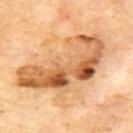About 11 mm across. A male subject aged around 70. The lesion-visualizer software estimated an average lesion color of about L≈61 a*≈22 b*≈41 (CIELAB) and roughly 15 lightness units darker than nearby skin. The software also gave a border-irregularity index near 6.5/10, a within-lesion color-variation index near 10/10, and a peripheral color-asymmetry measure near 4.5. The analysis additionally found a nevus-likeness score of about 25/100 and a detector confidence of about 55 out of 100 that the crop contains a lesion. This image is a 15 mm lesion crop taken from a total-body photograph. From the mid back.